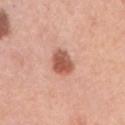Q: Was a biopsy performed?
A: no biopsy performed (imaged during a skin exam)
Q: Lesion location?
A: the right upper arm
Q: How was the tile lit?
A: white-light illumination
Q: What is the lesion's diameter?
A: about 3 mm
Q: What are the patient's age and sex?
A: female, in their 60s
Q: Automated lesion metrics?
A: an area of roughly 6.5 mm², an outline eccentricity of about 0.45 (0 = round, 1 = elongated), and two-axis asymmetry of about 0.2; a mean CIELAB color near L≈56 a*≈27 b*≈30 and a normalized border contrast of about 9.5
Q: What is the imaging modality?
A: 15 mm crop, total-body photography